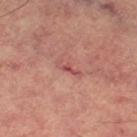This lesion was catalogued during total-body skin photography and was not selected for biopsy. The total-body-photography lesion software estimated an area of roughly 2.5 mm², an outline eccentricity of about 0.95 (0 = round, 1 = elongated), and two-axis asymmetry of about 0.4. The software also gave a lesion color around L≈50 a*≈29 b*≈24 in CIELAB, about 9 CIELAB-L* units darker than the surrounding skin, and a normalized border contrast of about 6.5. The software also gave an automated nevus-likeness rating near 0 out of 100. Longest diameter approximately 3 mm. This is a cross-polarized tile. A 15 mm close-up extracted from a 3D total-body photography capture. On the left thigh. A male patient aged 63 to 67.